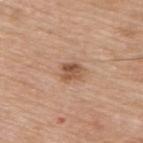Captured during whole-body skin photography for melanoma surveillance; the lesion was not biopsied.
From the back.
The recorded lesion diameter is about 2.5 mm.
A lesion tile, about 15 mm wide, cut from a 3D total-body photograph.
Imaged with white-light lighting.
A male subject approximately 75 years of age.